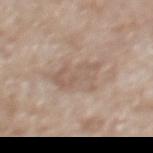Impression: The lesion was photographed on a routine skin check and not biopsied; there is no pathology result. Background: The lesion-visualizer software estimated a mean CIELAB color near L≈58 a*≈15 b*≈25, a lesion–skin lightness drop of about 7, and a lesion-to-skin contrast of about 5 (normalized; higher = more distinct). And it measured internal color variation of about 3 on a 0–10 scale. This image is a 15 mm lesion crop taken from a total-body photograph. A male patient about 65 years old. The lesion's longest dimension is about 5 mm. The tile uses white-light illumination. The lesion is located on the mid back.